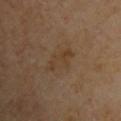Assessment: Recorded during total-body skin imaging; not selected for excision or biopsy. Acquisition and patient details: On the upper back. A roughly 15 mm field-of-view crop from a total-body skin photograph. The patient is a male aged around 70.